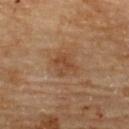The lesion was photographed on a routine skin check and not biopsied; there is no pathology result. The subject is a male aged around 85 or older. Automated image analysis of the tile measured a border-irregularity rating of about 2.5/10, internal color variation of about 3.5 on a 0–10 scale, and peripheral color asymmetry of about 1. On the upper back. The recorded lesion diameter is about 2.5 mm. Captured under cross-polarized illumination. A 15 mm close-up tile from a total-body photography series done for melanoma screening.